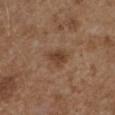Impression:
This lesion was catalogued during total-body skin photography and was not selected for biopsy.
Clinical summary:
A female subject approximately 35 years of age. Approximately 3.5 mm at its widest. A region of skin cropped from a whole-body photographic capture, roughly 15 mm wide. Captured under white-light illumination. The lesion is located on the right upper arm.